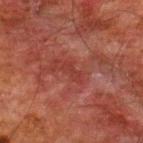follow-up = imaged on a skin check; not biopsied | subject = male, aged 58 to 62 | anatomic site = the upper back | acquisition = total-body-photography crop, ~15 mm field of view | TBP lesion metrics = a lesion color around L≈29 a*≈25 b*≈23 in CIELAB, a lesion–skin lightness drop of about 5, and a normalized border contrast of about 5; a within-lesion color-variation index near 0/10 | lesion size = ≈2.5 mm.